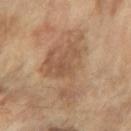Findings:
• follow-up — imaged on a skin check; not biopsied
• lesion size — ≈8.5 mm
• automated lesion analysis — a footprint of about 24 mm², an outline eccentricity of about 0.85 (0 = round, 1 = elongated), and a shape-asymmetry score of about 0.6 (0 = symmetric); about 8 CIELAB-L* units darker than the surrounding skin and a lesion-to-skin contrast of about 6 (normalized; higher = more distinct); border irregularity of about 8.5 on a 0–10 scale and a peripheral color-asymmetry measure near 1; an automated nevus-likeness rating near 0 out of 100 and lesion-presence confidence of about 100/100
• body site — the right forearm
• patient — female, aged approximately 70
• image — ~15 mm crop, total-body skin-cancer survey
• illumination — cross-polarized illumination